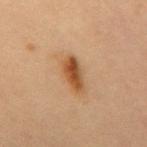Measured at roughly 4 mm in maximum diameter. Automated image analysis of the tile measured a shape-asymmetry score of about 0.25 (0 = symmetric). It also reported a classifier nevus-likeness of about 100/100 and a detector confidence of about 100 out of 100 that the crop contains a lesion. A female subject, aged 68 to 72. The tile uses cross-polarized illumination. A 15 mm crop from a total-body photograph taken for skin-cancer surveillance. On the mid back.